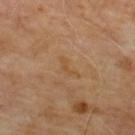Notes:
• biopsy status — total-body-photography surveillance lesion; no biopsy
• patient — male, aged approximately 65
• body site — the back
• acquisition — total-body-photography crop, ~15 mm field of view
• lesion size — ≈2.5 mm
• TBP lesion metrics — a lesion-to-skin contrast of about 5 (normalized; higher = more distinct); a border-irregularity index near 5.5/10; lesion-presence confidence of about 100/100